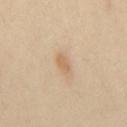Q: Was this lesion biopsied?
A: no biopsy performed (imaged during a skin exam)
Q: What is the imaging modality?
A: 15 mm crop, total-body photography
Q: How large is the lesion?
A: ≈2.5 mm
Q: How was the tile lit?
A: cross-polarized illumination
Q: Lesion location?
A: the mid back
Q: What are the patient's age and sex?
A: male, aged approximately 30